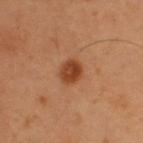Q: Was this lesion biopsied?
A: no biopsy performed (imaged during a skin exam)
Q: How was this image acquired?
A: ~15 mm crop, total-body skin-cancer survey
Q: How was the tile lit?
A: cross-polarized illumination
Q: What are the patient's age and sex?
A: male, roughly 55 years of age
Q: Lesion location?
A: the upper back